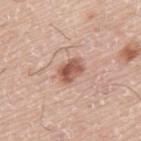The lesion is on the back. The lesion's longest dimension is about 3 mm. A male patient aged around 75. Automated image analysis of the tile measured a mean CIELAB color near L≈55 a*≈23 b*≈28, roughly 14 lightness units darker than nearby skin, and a normalized border contrast of about 9. It also reported a border-irregularity rating of about 2/10, a within-lesion color-variation index near 5.5/10, and radial color variation of about 2. The analysis additionally found a nevus-likeness score of about 90/100 and a detector confidence of about 100 out of 100 that the crop contains a lesion. Captured under white-light illumination. A roughly 15 mm field-of-view crop from a total-body skin photograph.